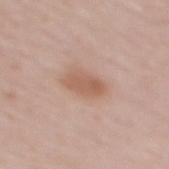Clinical impression:
Imaged during a routine full-body skin examination; the lesion was not biopsied and no histopathology is available.
Background:
From the mid back. The tile uses white-light illumination. The subject is a female aged around 60. Approximately 4 mm at its widest. Cropped from a total-body skin-imaging series; the visible field is about 15 mm.